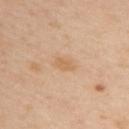• biopsy status: imaged on a skin check; not biopsied
• illumination: cross-polarized
• body site: the upper back
• patient: female, roughly 40 years of age
• lesion size: ≈2.5 mm
• acquisition: 15 mm crop, total-body photography